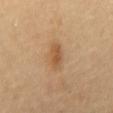notes: no biopsy performed (imaged during a skin exam); anatomic site: the front of the torso; acquisition: total-body-photography crop, ~15 mm field of view; subject: male, in their mid- to late 60s; lesion diameter: about 3.5 mm.